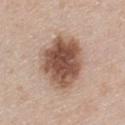| feature | finding |
|---|---|
| notes | catalogued during a skin exam; not biopsied |
| imaging modality | ~15 mm tile from a whole-body skin photo |
| subject | male, in their 40s |
| anatomic site | the upper back |